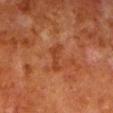Imaged during a routine full-body skin examination; the lesion was not biopsied and no histopathology is available. On the left lower leg. Imaged with cross-polarized lighting. A close-up tile cropped from a whole-body skin photograph, about 15 mm across. The patient is a male approximately 80 years of age. Measured at roughly 3.5 mm in maximum diameter.